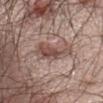Q: Lesion size?
A: ~5 mm (longest diameter)
Q: Who is the patient?
A: male, in their 50s
Q: Where on the body is the lesion?
A: the chest
Q: Illumination type?
A: white-light illumination
Q: How was this image acquired?
A: 15 mm crop, total-body photography